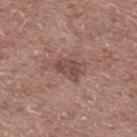{"biopsy_status": "not biopsied; imaged during a skin examination", "image": {"source": "total-body photography crop", "field_of_view_mm": 15}, "site": "back", "lighting": "white-light", "patient": {"sex": "male", "age_approx": 60}, "automated_metrics": {"area_mm2_approx": 5.0, "color_variation_0_10": 3.0, "peripheral_color_asymmetry": 1.0, "nevus_likeness_0_100": 0, "lesion_detection_confidence_0_100": 100}}